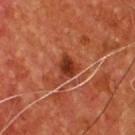biopsy status — total-body-photography surveillance lesion; no biopsy
image source — ~15 mm tile from a whole-body skin photo
patient — male, aged approximately 55
diameter — about 3 mm
location — the chest
lighting — cross-polarized illumination